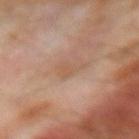Imaged during a routine full-body skin examination; the lesion was not biopsied and no histopathology is available. From the right forearm. A lesion tile, about 15 mm wide, cut from a 3D total-body photograph. An algorithmic analysis of the crop reported an eccentricity of roughly 0.85. The software also gave a lesion–skin lightness drop of about 6 and a normalized border contrast of about 5. The analysis additionally found a nevus-likeness score of about 0/100. A male subject aged approximately 70.